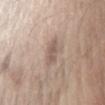{"biopsy_status": "not biopsied; imaged during a skin examination", "image": {"source": "total-body photography crop", "field_of_view_mm": 15}, "lighting": "white-light", "patient": {"sex": "male", "age_approx": 70}, "site": "left forearm", "lesion_size": {"long_diameter_mm_approx": 2.5}}